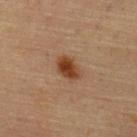workup: imaged on a skin check; not biopsied | image source: ~15 mm crop, total-body skin-cancer survey | site: the upper back | automated lesion analysis: two-axis asymmetry of about 0.15; a border-irregularity rating of about 1.5/10 and peripheral color asymmetry of about 1 | subject: female, about 65 years old | tile lighting: cross-polarized illumination.